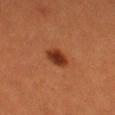workup=imaged on a skin check; not biopsied | TBP lesion metrics=an eccentricity of roughly 0.8 and two-axis asymmetry of about 0.25; a lesion–skin lightness drop of about 12 and a normalized border contrast of about 10.5; a border-irregularity rating of about 2/10, internal color variation of about 3 on a 0–10 scale, and a peripheral color-asymmetry measure near 1 | lighting=cross-polarized illumination | image=15 mm crop, total-body photography | anatomic site=the leg | size=about 3.5 mm | subject=female, roughly 30 years of age.